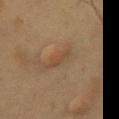Clinical impression:
Recorded during total-body skin imaging; not selected for excision or biopsy.
Image and clinical context:
A 15 mm crop from a total-body photograph taken for skin-cancer surveillance. A male patient, aged 48 to 52. Located on the chest.